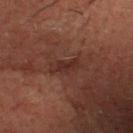| field | value |
|---|---|
| notes | catalogued during a skin exam; not biopsied |